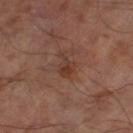No biopsy was performed on this lesion — it was imaged during a full skin examination and was not determined to be concerning.
A male subject, aged around 65.
Imaged with cross-polarized lighting.
About 3 mm across.
A close-up tile cropped from a whole-body skin photograph, about 15 mm across.
On the left thigh.